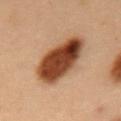The lesion was tiled from a total-body skin photograph and was not biopsied. A roughly 15 mm field-of-view crop from a total-body skin photograph. The subject is a male in their mid-50s. On the abdomen.